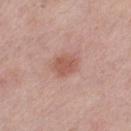Q: Was a biopsy performed?
A: imaged on a skin check; not biopsied
Q: Lesion location?
A: the left thigh
Q: How was the tile lit?
A: white-light
Q: Automated lesion metrics?
A: a footprint of about 5.5 mm², an eccentricity of roughly 0.5, and two-axis asymmetry of about 0.2; an average lesion color of about L≈56 a*≈23 b*≈28 (CIELAB) and roughly 9 lightness units darker than nearby skin; a detector confidence of about 100 out of 100 that the crop contains a lesion
Q: How large is the lesion?
A: ≈2.5 mm
Q: What are the patient's age and sex?
A: female, aged 38 to 42
Q: How was this image acquired?
A: ~15 mm tile from a whole-body skin photo